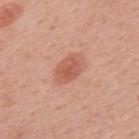<record>
  <biopsy_status>not biopsied; imaged during a skin examination</biopsy_status>
</record>